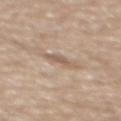{
  "biopsy_status": "not biopsied; imaged during a skin examination",
  "patient": {
    "sex": "male",
    "age_approx": 70
  },
  "lighting": "white-light",
  "lesion_size": {
    "long_diameter_mm_approx": 3.0
  },
  "automated_metrics": {
    "border_irregularity_0_10": 3.5,
    "color_variation_0_10": 0.5,
    "peripheral_color_asymmetry": 0.0,
    "nevus_likeness_0_100": 0,
    "lesion_detection_confidence_0_100": 100
  },
  "site": "mid back",
  "image": {
    "source": "total-body photography crop",
    "field_of_view_mm": 15
  }
}